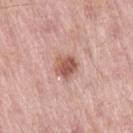notes — catalogued during a skin exam; not biopsied
illumination — white-light illumination
patient — male, aged around 55
body site — the right thigh
size — ~3 mm (longest diameter)
imaging modality — 15 mm crop, total-body photography